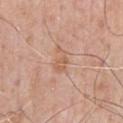Background:
From the chest. A 15 mm crop from a total-body photograph taken for skin-cancer surveillance. A male subject, aged 73–77.